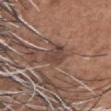This lesion was catalogued during total-body skin photography and was not selected for biopsy. A male subject, aged 63 to 67. A close-up tile cropped from a whole-body skin photograph, about 15 mm across. An algorithmic analysis of the crop reported a lesion area of about 5 mm², an eccentricity of roughly 0.65, and a shape-asymmetry score of about 0.35 (0 = symmetric). And it measured a mean CIELAB color near L≈42 a*≈18 b*≈22, about 9 CIELAB-L* units darker than the surrounding skin, and a lesion-to-skin contrast of about 8 (normalized; higher = more distinct). And it measured a classifier nevus-likeness of about 0/100 and lesion-presence confidence of about 70/100. On the head or neck. Imaged with white-light lighting.